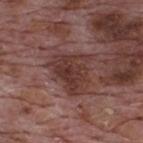{"biopsy_status": "not biopsied; imaged during a skin examination", "lighting": "white-light", "image": {"source": "total-body photography crop", "field_of_view_mm": 15}, "automated_metrics": {"cielab_L": 36, "cielab_a": 20, "cielab_b": 22, "vs_skin_darker_L": 10.0, "border_irregularity_0_10": 4.5, "color_variation_0_10": 4.5, "peripheral_color_asymmetry": 1.5}, "patient": {"sex": "male", "age_approx": 70}, "lesion_size": {"long_diameter_mm_approx": 5.0}, "site": "upper back"}